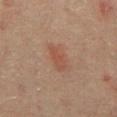No biopsy was performed on this lesion — it was imaged during a full skin examination and was not determined to be concerning.
On the abdomen.
A male patient, aged 63 to 67.
Cropped from a total-body skin-imaging series; the visible field is about 15 mm.